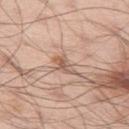Assessment:
Part of a total-body skin-imaging series; this lesion was reviewed on a skin check and was not flagged for biopsy.
Image and clinical context:
Imaged with white-light lighting. A 15 mm close-up tile from a total-body photography series done for melanoma screening. Longest diameter approximately 3 mm. An algorithmic analysis of the crop reported an area of roughly 4 mm². And it measured a lesion color around L≈59 a*≈19 b*≈28 in CIELAB, roughly 9 lightness units darker than nearby skin, and a normalized lesion–skin contrast near 6. And it measured a border-irregularity index near 4/10, a within-lesion color-variation index near 5.5/10, and radial color variation of about 1.5. The subject is a male roughly 55 years of age. The lesion is located on the left thigh.